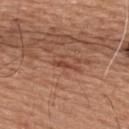This lesion was catalogued during total-body skin photography and was not selected for biopsy. Captured under white-light illumination. A male patient roughly 65 years of age. About 3 mm across. This image is a 15 mm lesion crop taken from a total-body photograph. From the back.